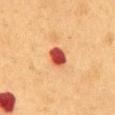| field | value |
|---|---|
| notes | total-body-photography surveillance lesion; no biopsy |
| tile lighting | cross-polarized illumination |
| subject | male, aged around 55 |
| anatomic site | the front of the torso |
| image | 15 mm crop, total-body photography |
| automated metrics | about 20 CIELAB-L* units darker than the surrounding skin and a lesion-to-skin contrast of about 13.5 (normalized; higher = more distinct); a detector confidence of about 100 out of 100 that the crop contains a lesion |